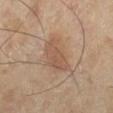biopsy status=catalogued during a skin exam; not biopsied
patient=female, approximately 70 years of age
location=the left lower leg
lesion diameter=about 5 mm
imaging modality=~15 mm tile from a whole-body skin photo